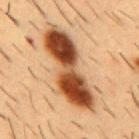Impression: Imaged during a routine full-body skin examination; the lesion was not biopsied and no histopathology is available. Acquisition and patient details: Measured at roughly 10.5 mm in maximum diameter. This image is a 15 mm lesion crop taken from a total-body photograph. The patient is a male aged approximately 55. The lesion is located on the chest.